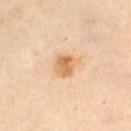Q: Was this lesion biopsied?
A: catalogued during a skin exam; not biopsied